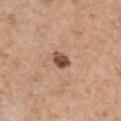Impression: Imaged during a routine full-body skin examination; the lesion was not biopsied and no histopathology is available. Clinical summary: The lesion-visualizer software estimated a mean CIELAB color near L≈49 a*≈21 b*≈29, a lesion–skin lightness drop of about 16, and a normalized border contrast of about 11. The analysis additionally found a border-irregularity rating of about 1.5/10, a within-lesion color-variation index near 6/10, and a peripheral color-asymmetry measure near 2. A roughly 15 mm field-of-view crop from a total-body skin photograph. The lesion is on the front of the torso. A male subject, aged approximately 60. Longest diameter approximately 2.5 mm.